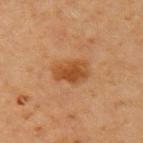workup — imaged on a skin check; not biopsied | image source — ~15 mm crop, total-body skin-cancer survey | subject — male, aged around 70 | automated metrics — an average lesion color of about L≈40 a*≈21 b*≈34 (CIELAB) and a normalized border contrast of about 8; an automated nevus-likeness rating near 90 out of 100 and a lesion-detection confidence of about 100/100 | tile lighting — cross-polarized | anatomic site — the right upper arm.